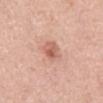Notes:
• follow-up: no biopsy performed (imaged during a skin exam)
• subject: male, aged approximately 65
• image: ~15 mm crop, total-body skin-cancer survey
• site: the abdomen
• illumination: white-light illumination